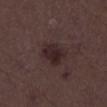Assessment: Imaged during a routine full-body skin examination; the lesion was not biopsied and no histopathology is available. Background: The patient is a male roughly 50 years of age. A region of skin cropped from a whole-body photographic capture, roughly 15 mm wide.